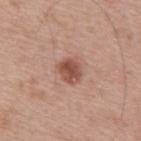The lesion was photographed on a routine skin check and not biopsied; there is no pathology result. The tile uses white-light illumination. Measured at roughly 3 mm in maximum diameter. The lesion is located on the mid back. Cropped from a whole-body photographic skin survey; the tile spans about 15 mm. The patient is a male aged approximately 55.